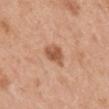notes=imaged on a skin check; not biopsied | acquisition=total-body-photography crop, ~15 mm field of view | location=the back | subject=male, aged approximately 30.